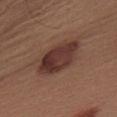Captured during whole-body skin photography for melanoma surveillance; the lesion was not biopsied. Imaged with white-light lighting. A 15 mm crop from a total-body photograph taken for skin-cancer surveillance. The subject is a male roughly 55 years of age. On the upper back.